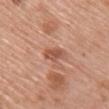Q: Was a biopsy performed?
A: catalogued during a skin exam; not biopsied
Q: How was this image acquired?
A: total-body-photography crop, ~15 mm field of view
Q: What are the patient's age and sex?
A: female, aged approximately 65
Q: How large is the lesion?
A: ~3 mm (longest diameter)
Q: Where on the body is the lesion?
A: the back